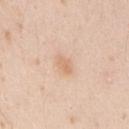Clinical impression: Captured during whole-body skin photography for melanoma surveillance; the lesion was not biopsied. Context: A male patient in their mid- to late 20s. The tile uses white-light illumination. A 15 mm crop from a total-body photograph taken for skin-cancer surveillance. From the right upper arm.